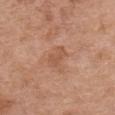biopsy status: imaged on a skin check; not biopsied
imaging modality: total-body-photography crop, ~15 mm field of view
subject: female, approximately 65 years of age
image-analysis metrics: a lesion area of about 3.5 mm², an outline eccentricity of about 0.85 (0 = round, 1 = elongated), and a shape-asymmetry score of about 0.45 (0 = symmetric)
lesion size: ~3 mm (longest diameter)
location: the chest
illumination: white-light illumination